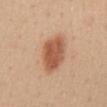Clinical impression: Recorded during total-body skin imaging; not selected for excision or biopsy. Clinical summary: A 15 mm crop from a total-body photograph taken for skin-cancer surveillance. A female patient aged 43–47. Automated tile analysis of the lesion measured a classifier nevus-likeness of about 100/100 and lesion-presence confidence of about 100/100. The lesion is on the chest. This is a white-light tile.